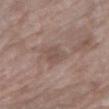Findings:
- biopsy status · no biopsy performed (imaged during a skin exam)
- patient · female, aged approximately 70
- diameter · ~3.5 mm (longest diameter)
- image · ~15 mm tile from a whole-body skin photo
- lighting · white-light illumination
- body site · the right upper arm